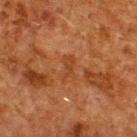Part of a total-body skin-imaging series; this lesion was reviewed on a skin check and was not flagged for biopsy.
The patient is a male aged around 60.
Automated image analysis of the tile measured a lesion area of about 4 mm², an outline eccentricity of about 0.85 (0 = round, 1 = elongated), and two-axis asymmetry of about 0.45. And it measured an average lesion color of about L≈33 a*≈22 b*≈31 (CIELAB), about 5 CIELAB-L* units darker than the surrounding skin, and a normalized border contrast of about 5.5. The analysis additionally found border irregularity of about 5 on a 0–10 scale and internal color variation of about 0.5 on a 0–10 scale. It also reported a classifier nevus-likeness of about 0/100.
Imaged with cross-polarized lighting.
The lesion is located on the upper back.
Longest diameter approximately 3 mm.
A 15 mm close-up tile from a total-body photography series done for melanoma screening.